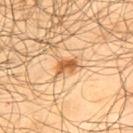This lesion was catalogued during total-body skin photography and was not selected for biopsy. Imaged with cross-polarized lighting. The lesion's longest dimension is about 3 mm. A male subject, approximately 65 years of age. The lesion is on the back. Cropped from a total-body skin-imaging series; the visible field is about 15 mm.